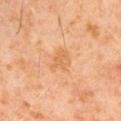Part of a total-body skin-imaging series; this lesion was reviewed on a skin check and was not flagged for biopsy.
A male patient, aged around 60.
On the left upper arm.
Cropped from a whole-body photographic skin survey; the tile spans about 15 mm.
Captured under cross-polarized illumination.